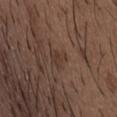Q: Is there a histopathology result?
A: catalogued during a skin exam; not biopsied
Q: Where on the body is the lesion?
A: the chest
Q: What is the imaging modality?
A: ~15 mm crop, total-body skin-cancer survey
Q: Who is the patient?
A: male, about 50 years old